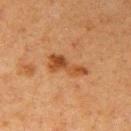Case summary:
- follow-up: total-body-photography surveillance lesion; no biopsy
- imaging modality: total-body-photography crop, ~15 mm field of view
- lighting: cross-polarized illumination
- lesion diameter: ~5 mm (longest diameter)
- automated metrics: an area of roughly 7.5 mm² and a symmetry-axis asymmetry near 0.4; a mean CIELAB color near L≈41 a*≈22 b*≈35 and a normalized lesion–skin contrast near 8
- location: the right upper arm
- subject: female, aged around 40